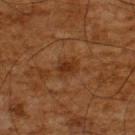* subject: male, about 65 years old
* site: the upper back
* acquisition: 15 mm crop, total-body photography